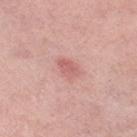<case>
<biopsy_status>not biopsied; imaged during a skin examination</biopsy_status>
<patient>
  <sex>female</sex>
  <age_approx>50</age_approx>
</patient>
<lighting>white-light</lighting>
<site>left thigh</site>
<image>
  <source>total-body photography crop</source>
  <field_of_view_mm>15</field_of_view_mm>
</image>
<lesion_size>
  <long_diameter_mm_approx>3.0</long_diameter_mm_approx>
</lesion_size>
</case>